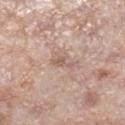  biopsy_status: not biopsied; imaged during a skin examination
  patient:
    sex: female
    age_approx: 70
  automated_metrics:
    border_irregularity_0_10: 5.5
    color_variation_0_10: 0.0
    peripheral_color_asymmetry: 0.0
    nevus_likeness_0_100: 0
    lesion_detection_confidence_0_100: 100
  lesion_size:
    long_diameter_mm_approx: 3.0
  image:
    source: total-body photography crop
    field_of_view_mm: 15
  site: right lower leg
  lighting: white-light